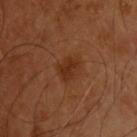Imaged during a routine full-body skin examination; the lesion was not biopsied and no histopathology is available. Located on the back. This is a cross-polarized tile. Cropped from a whole-body photographic skin survey; the tile spans about 15 mm. The subject is a male aged around 55.